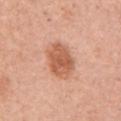The lesion was tiled from a total-body skin photograph and was not biopsied. About 4.5 mm across. The lesion is located on the left upper arm. A female subject, aged 58 to 62. A 15 mm close-up extracted from a 3D total-body photography capture. The total-body-photography lesion software estimated an outline eccentricity of about 0.7 (0 = round, 1 = elongated) and two-axis asymmetry of about 0.15. The software also gave a border-irregularity index near 2/10 and internal color variation of about 3.5 on a 0–10 scale. The software also gave lesion-presence confidence of about 100/100.